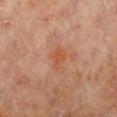Assessment: The lesion was tiled from a total-body skin photograph and was not biopsied. Image and clinical context: The lesion is on the right lower leg. Imaged with cross-polarized lighting. A female patient, aged 78 to 82. A roughly 15 mm field-of-view crop from a total-body skin photograph. The lesion's longest dimension is about 2.5 mm.